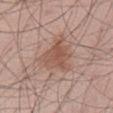From the front of the torso.
This is a white-light tile.
A male patient, in their mid-50s.
A 15 mm close-up tile from a total-body photography series done for melanoma screening.
Longest diameter approximately 4.5 mm.